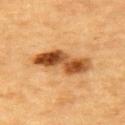Q: Is there a histopathology result?
A: catalogued during a skin exam; not biopsied
Q: How was this image acquired?
A: ~15 mm tile from a whole-body skin photo
Q: Where on the body is the lesion?
A: the upper back
Q: Who is the patient?
A: male, about 85 years old or older